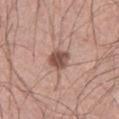* biopsy status: catalogued during a skin exam; not biopsied
* patient: male, roughly 40 years of age
* image: 15 mm crop, total-body photography
* size: ~2.5 mm (longest diameter)
* lighting: white-light
* location: the leg
* automated metrics: an average lesion color of about L≈51 a*≈20 b*≈24 (CIELAB), roughly 14 lightness units darker than nearby skin, and a normalized border contrast of about 10; border irregularity of about 1.5 on a 0–10 scale and radial color variation of about 1; a classifier nevus-likeness of about 90/100